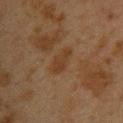| key | value |
|---|---|
| biopsy status | catalogued during a skin exam; not biopsied |
| location | the back |
| imaging modality | 15 mm crop, total-body photography |
| diameter | ~4 mm (longest diameter) |
| lighting | cross-polarized illumination |
| patient | female, roughly 40 years of age |